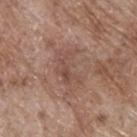follow-up: total-body-photography surveillance lesion; no biopsy
TBP lesion metrics: an area of roughly 10 mm² and a shape-asymmetry score of about 0.4 (0 = symmetric); a classifier nevus-likeness of about 0/100
patient: male, aged 68 to 72
illumination: white-light
site: the back
size: ≈5 mm
image: ~15 mm crop, total-body skin-cancer survey